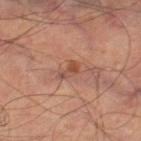Q: Was a biopsy performed?
A: total-body-photography surveillance lesion; no biopsy
Q: What is the anatomic site?
A: the right thigh
Q: Automated lesion metrics?
A: a lesion area of about 3 mm², an eccentricity of roughly 0.85, and two-axis asymmetry of about 0.45; a lesion color around L≈51 a*≈24 b*≈31 in CIELAB; a border-irregularity rating of about 4.5/10, a within-lesion color-variation index near 1.5/10, and a peripheral color-asymmetry measure near 0
Q: What kind of image is this?
A: ~15 mm tile from a whole-body skin photo
Q: How was the tile lit?
A: cross-polarized illumination
Q: Lesion size?
A: ≈2.5 mm